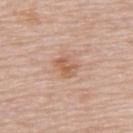Captured during whole-body skin photography for melanoma surveillance; the lesion was not biopsied.
Automated tile analysis of the lesion measured a nevus-likeness score of about 0/100 and a detector confidence of about 100 out of 100 that the crop contains a lesion.
The tile uses white-light illumination.
Cropped from a whole-body photographic skin survey; the tile spans about 15 mm.
The subject is a female in their mid- to late 60s.
Measured at roughly 3 mm in maximum diameter.
The lesion is on the upper back.